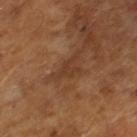follow-up = no biopsy performed (imaged during a skin exam)
patient = male, roughly 65 years of age
image source = 15 mm crop, total-body photography